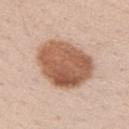{
  "biopsy_status": "not biopsied; imaged during a skin examination",
  "site": "right upper arm",
  "patient": {
    "sex": "female",
    "age_approx": 40
  },
  "lesion_size": {
    "long_diameter_mm_approx": 7.0
  },
  "lighting": "white-light",
  "automated_metrics": {
    "cielab_L": 57,
    "cielab_a": 21,
    "cielab_b": 31,
    "nevus_likeness_0_100": 40
  },
  "image": {
    "source": "total-body photography crop",
    "field_of_view_mm": 15
  }
}